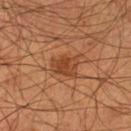acquisition = total-body-photography crop, ~15 mm field of view; subject = male, roughly 55 years of age; lesion size = ≈3.5 mm; location = the left lower leg.